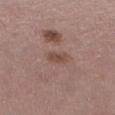Impression: No biopsy was performed on this lesion — it was imaged during a full skin examination and was not determined to be concerning. Clinical summary: Measured at roughly 2.5 mm in maximum diameter. A female patient, about 40 years old. The total-body-photography lesion software estimated a lesion color around L≈47 a*≈20 b*≈24 in CIELAB and a normalized lesion–skin contrast near 6.5. Imaged with white-light lighting. On the left thigh. A close-up tile cropped from a whole-body skin photograph, about 15 mm across.